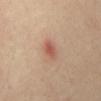Q: Is there a histopathology result?
A: imaged on a skin check; not biopsied
Q: What is the anatomic site?
A: the mid back
Q: What lighting was used for the tile?
A: cross-polarized illumination
Q: What kind of image is this?
A: total-body-photography crop, ~15 mm field of view
Q: Lesion size?
A: about 2.5 mm
Q: What are the patient's age and sex?
A: female, about 40 years old
Q: What did automated image analysis measure?
A: a footprint of about 4 mm² and two-axis asymmetry of about 0.15; a nevus-likeness score of about 10/100 and lesion-presence confidence of about 100/100